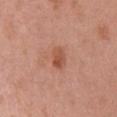follow-up: catalogued during a skin exam; not biopsied
image source: total-body-photography crop, ~15 mm field of view
lesion size: ~2.5 mm (longest diameter)
site: the right upper arm
tile lighting: white-light illumination
automated lesion analysis: an area of roughly 4.5 mm² and an eccentricity of roughly 0.75; an average lesion color of about L≈53 a*≈26 b*≈32 (CIELAB) and a lesion-to-skin contrast of about 7 (normalized; higher = more distinct); a classifier nevus-likeness of about 35/100 and lesion-presence confidence of about 100/100
patient: female, about 40 years old